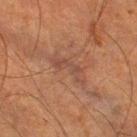{
  "biopsy_status": "not biopsied; imaged during a skin examination",
  "lighting": "cross-polarized",
  "image": {
    "source": "total-body photography crop",
    "field_of_view_mm": 15
  },
  "automated_metrics": {
    "area_mm2_approx": 4.5,
    "eccentricity": 0.95,
    "cielab_L": 37,
    "cielab_a": 18,
    "cielab_b": 23,
    "vs_skin_contrast_norm": 5.5,
    "border_irregularity_0_10": 8.0,
    "color_variation_0_10": 1.0,
    "peripheral_color_asymmetry": 0.0,
    "nevus_likeness_0_100": 0,
    "lesion_detection_confidence_0_100": 60
  },
  "patient": {
    "sex": "male",
    "age_approx": 65
  },
  "lesion_size": {
    "long_diameter_mm_approx": 4.5
  },
  "site": "right lower leg"
}